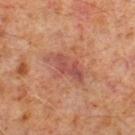Q: Was this lesion biopsied?
A: catalogued during a skin exam; not biopsied
Q: What is the lesion's diameter?
A: about 5 mm
Q: How was the tile lit?
A: cross-polarized illumination
Q: Patient demographics?
A: male, aged 58–62
Q: Where on the body is the lesion?
A: the right lower leg
Q: What did automated image analysis measure?
A: roughly 8 lightness units darker than nearby skin
Q: What is the imaging modality?
A: total-body-photography crop, ~15 mm field of view